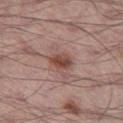patient=male, roughly 70 years of age
location=the leg
lighting=white-light illumination
image=~15 mm tile from a whole-body skin photo
automated metrics=a classifier nevus-likeness of about 90/100 and lesion-presence confidence of about 100/100